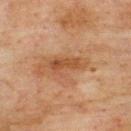| field | value |
|---|---|
| workup | total-body-photography surveillance lesion; no biopsy |
| site | the back |
| subject | male, aged around 75 |
| lesion size | ~5 mm (longest diameter) |
| tile lighting | cross-polarized illumination |
| TBP lesion metrics | a footprint of about 7.5 mm², an eccentricity of roughly 0.9, and a shape-asymmetry score of about 0.4 (0 = symmetric) |
| image | total-body-photography crop, ~15 mm field of view |